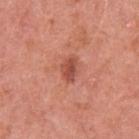| feature | finding |
|---|---|
| patient | male, roughly 55 years of age |
| lighting | white-light illumination |
| imaging modality | ~15 mm crop, total-body skin-cancer survey |
| diameter | ≈3 mm |
| location | the left upper arm |
| automated lesion analysis | a lesion color around L≈51 a*≈30 b*≈31 in CIELAB, roughly 11 lightness units darker than nearby skin, and a normalized lesion–skin contrast near 7.5; a border-irregularity rating of about 2/10, a color-variation rating of about 3/10, and peripheral color asymmetry of about 1; a nevus-likeness score of about 40/100 and lesion-presence confidence of about 100/100 |